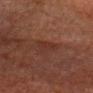| field | value |
|---|---|
| follow-up | total-body-photography surveillance lesion; no biopsy |
| lesion size | ~3.5 mm (longest diameter) |
| subject | male, about 80 years old |
| illumination | cross-polarized illumination |
| anatomic site | the head or neck |
| image source | total-body-photography crop, ~15 mm field of view |